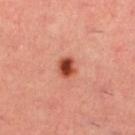Assessment:
The lesion was tiled from a total-body skin photograph and was not biopsied.
Image and clinical context:
The lesion's longest dimension is about 2.5 mm. On the left lower leg. Cropped from a whole-body photographic skin survey; the tile spans about 15 mm. The subject is a female approximately 35 years of age. Automated tile analysis of the lesion measured an area of roughly 4.5 mm², an outline eccentricity of about 0.55 (0 = round, 1 = elongated), and two-axis asymmetry of about 0.2. It also reported a normalized lesion–skin contrast near 11.5. The analysis additionally found a border-irregularity index near 1.5/10, internal color variation of about 4.5 on a 0–10 scale, and radial color variation of about 1.5. The analysis additionally found a classifier nevus-likeness of about 100/100 and lesion-presence confidence of about 100/100.